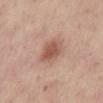  site: abdomen
  patient:
    sex: male
    age_approx: 55
  lighting: white-light
  lesion_size:
    long_diameter_mm_approx: 3.5
  image:
    source: total-body photography crop
    field_of_view_mm: 15
  automated_metrics:
    eccentricity: 0.65
    shape_asymmetry: 0.2
    vs_skin_darker_L: 11.0
    vs_skin_contrast_norm: 7.5